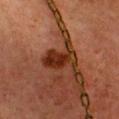biopsy_status: not biopsied; imaged during a skin examination
lesion_size:
  long_diameter_mm_approx: 4.5
lighting: cross-polarized
site: chest
image:
  source: total-body photography crop
  field_of_view_mm: 15
automated_metrics:
  cielab_L: 25
  cielab_a: 23
  cielab_b: 28
  vs_skin_contrast_norm: 11.0
patient:
  sex: female
  age_approx: 40